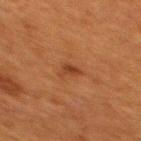{
  "biopsy_status": "not biopsied; imaged during a skin examination",
  "patient": {
    "sex": "female",
    "age_approx": 40
  },
  "site": "upper back",
  "image": {
    "source": "total-body photography crop",
    "field_of_view_mm": 15
  },
  "lighting": "cross-polarized",
  "lesion_size": {
    "long_diameter_mm_approx": 2.5
  }
}